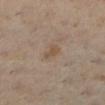image: 15 mm crop, total-body photography | patient: female, roughly 45 years of age | anatomic site: the left lower leg.